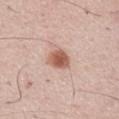Captured during whole-body skin photography for melanoma surveillance; the lesion was not biopsied. The lesion is located on the chest. A close-up tile cropped from a whole-body skin photograph, about 15 mm across. The subject is a male aged approximately 60. The tile uses white-light illumination. Measured at roughly 2.5 mm in maximum diameter. Automated tile analysis of the lesion measured a border-irregularity rating of about 2/10.